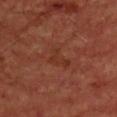- anatomic site: the chest
- image source: ~15 mm crop, total-body skin-cancer survey
- lesion size: about 3 mm
- tile lighting: cross-polarized
- subject: male, about 65 years old